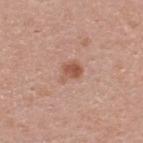Imaged during a routine full-body skin examination; the lesion was not biopsied and no histopathology is available.